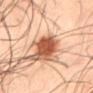Assessment:
No biopsy was performed on this lesion — it was imaged during a full skin examination and was not determined to be concerning.
Clinical summary:
Automated tile analysis of the lesion measured an area of roughly 7 mm², an outline eccentricity of about 0.9 (0 = round, 1 = elongated), and a symmetry-axis asymmetry near 0.2. And it measured an average lesion color of about L≈52 a*≈30 b*≈36 (CIELAB), roughly 20 lightness units darker than nearby skin, and a normalized border contrast of about 12.5. And it measured a border-irregularity rating of about 2.5/10, a within-lesion color-variation index near 4/10, and a peripheral color-asymmetry measure near 1.5. The analysis additionally found a detector confidence of about 100 out of 100 that the crop contains a lesion. A 15 mm close-up tile from a total-body photography series done for melanoma screening. A male patient in their 50s. About 4.5 mm across. This is a cross-polarized tile. The lesion is on the mid back.